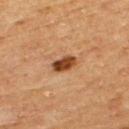Findings:
– notes — catalogued during a skin exam; not biopsied
– location — the upper back
– lighting — cross-polarized illumination
– imaging modality — ~15 mm tile from a whole-body skin photo
– subject — male, aged around 65